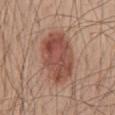Impression: The lesion was tiled from a total-body skin photograph and was not biopsied. Acquisition and patient details: Imaged with white-light lighting. A male subject, aged around 50. Longest diameter approximately 6.5 mm. A 15 mm crop from a total-body photograph taken for skin-cancer surveillance. The lesion-visualizer software estimated an average lesion color of about L≈49 a*≈23 b*≈27 (CIELAB), about 11 CIELAB-L* units darker than the surrounding skin, and a normalized lesion–skin contrast near 8.5. From the mid back.